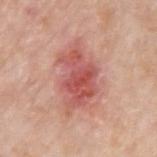The lesion was tiled from a total-body skin photograph and was not biopsied.
Located on the arm.
A 15 mm close-up tile from a total-body photography series done for melanoma screening.
A male subject, in their mid- to late 70s.